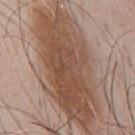Part of a total-body skin-imaging series; this lesion was reviewed on a skin check and was not flagged for biopsy. The subject is a male in their mid- to late 60s. Located on the chest. A close-up tile cropped from a whole-body skin photograph, about 15 mm across. Automated tile analysis of the lesion measured an area of roughly 65 mm², a shape eccentricity near 0.95, and a symmetry-axis asymmetry near 0.2. It also reported a normalized border contrast of about 9.5. The lesion's longest dimension is about 15 mm. This is a white-light tile.